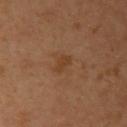Clinical impression:
This lesion was catalogued during total-body skin photography and was not selected for biopsy.
Acquisition and patient details:
Located on the right upper arm. A female patient in their 40s. Imaged with cross-polarized lighting. A close-up tile cropped from a whole-body skin photograph, about 15 mm across. Measured at roughly 2.5 mm in maximum diameter.